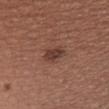Background: The lesion is on the chest. A lesion tile, about 15 mm wide, cut from a 3D total-body photograph. A male subject about 40 years old. This is a white-light tile.